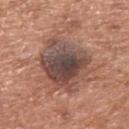workup = total-body-photography surveillance lesion; no biopsy
location = the upper back
size = ~8 mm (longest diameter)
tile lighting = white-light
image = 15 mm crop, total-body photography
subject = male, aged around 65
TBP lesion metrics = a color-variation rating of about 8.5/10 and a peripheral color-asymmetry measure near 2